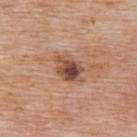follow-up=no biopsy performed (imaged during a skin exam) | lighting=white-light | subject=female, aged 73–77 | imaging modality=~15 mm tile from a whole-body skin photo | site=the upper back | diameter=about 4 mm.